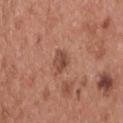Acquisition and patient details:
Captured under white-light illumination. The recorded lesion diameter is about 2.5 mm. A lesion tile, about 15 mm wide, cut from a 3D total-body photograph. The patient is a male aged around 55. From the back.